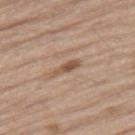This is a white-light tile.
On the leg.
The patient is a male aged 68 to 72.
Measured at roughly 3.5 mm in maximum diameter.
A 15 mm crop from a total-body photograph taken for skin-cancer surveillance.
Automated image analysis of the tile measured about 11 CIELAB-L* units darker than the surrounding skin and a normalized border contrast of about 8. And it measured a lesion-detection confidence of about 100/100.